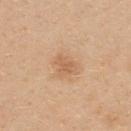| key | value |
|---|---|
| notes | catalogued during a skin exam; not biopsied |
| lighting | white-light illumination |
| subject | female, in their mid- to late 20s |
| anatomic site | the upper back |
| automated metrics | an average lesion color of about L≈61 a*≈21 b*≈37 (CIELAB), a lesion–skin lightness drop of about 8, and a normalized border contrast of about 5.5 |
| image | ~15 mm tile from a whole-body skin photo |